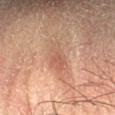Clinical summary:
A male subject approximately 60 years of age. The lesion is located on the right forearm. A region of skin cropped from a whole-body photographic capture, roughly 15 mm wide.